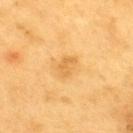<record>
<biopsy_status>not biopsied; imaged during a skin examination</biopsy_status>
<lighting>cross-polarized</lighting>
<automated_metrics>
  <area_mm2_approx>3.5</area_mm2_approx>
  <eccentricity>0.8</eccentricity>
  <border_irregularity_0_10>3.5</border_irregularity_0_10>
  <color_variation_0_10>1.5</color_variation_0_10>
  <lesion_detection_confidence_0_100>100</lesion_detection_confidence_0_100>
</automated_metrics>
<lesion_size>
  <long_diameter_mm_approx>3.0</long_diameter_mm_approx>
</lesion_size>
<site>upper back</site>
<image>
  <source>total-body photography crop</source>
  <field_of_view_mm>15</field_of_view_mm>
</image>
<patient>
  <sex>male</sex>
  <age_approx>60</age_approx>
</patient>
</record>